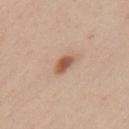Captured during whole-body skin photography for melanoma surveillance; the lesion was not biopsied. A female subject, aged approximately 40. Located on the mid back. Automated tile analysis of the lesion measured a within-lesion color-variation index near 3.5/10 and peripheral color asymmetry of about 1. And it measured a nevus-likeness score of about 95/100. A 15 mm crop from a total-body photograph taken for skin-cancer surveillance. About 3 mm across. This is a white-light tile.